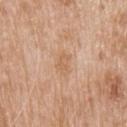Q: Was this lesion biopsied?
A: imaged on a skin check; not biopsied
Q: Lesion location?
A: the head or neck
Q: Patient demographics?
A: male, approximately 80 years of age
Q: How large is the lesion?
A: ~3 mm (longest diameter)
Q: How was this image acquired?
A: total-body-photography crop, ~15 mm field of view